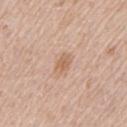The lesion was photographed on a routine skin check and not biopsied; there is no pathology result. The recorded lesion diameter is about 3 mm. A male subject, aged approximately 65. This image is a 15 mm lesion crop taken from a total-body photograph. On the left upper arm.